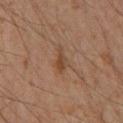<record>
  <biopsy_status>not biopsied; imaged during a skin examination</biopsy_status>
  <image>
    <source>total-body photography crop</source>
    <field_of_view_mm>15</field_of_view_mm>
  </image>
  <lesion_size>
    <long_diameter_mm_approx>3.0</long_diameter_mm_approx>
  </lesion_size>
  <lighting>cross-polarized</lighting>
  <patient>
    <sex>male</sex>
    <age_approx>45</age_approx>
  </patient>
  <site>left lower leg</site>
  <automated_metrics>
    <cielab_L>34</cielab_L>
    <cielab_a>16</cielab_a>
    <cielab_b>26</cielab_b>
    <vs_skin_darker_L>6.0</vs_skin_darker_L>
    <vs_skin_contrast_norm>6.5</vs_skin_contrast_norm>
    <border_irregularity_0_10>3.0</border_irregularity_0_10>
    <peripheral_color_asymmetry>1.0</peripheral_color_asymmetry>
    <nevus_likeness_0_100>10</nevus_likeness_0_100>
    <lesion_detection_confidence_0_100>100</lesion_detection_confidence_0_100>
  </automated_metrics>
</record>